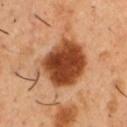Clinical impression: Imaged during a routine full-body skin examination; the lesion was not biopsied and no histopathology is available. Image and clinical context: The recorded lesion diameter is about 6.5 mm. Imaged with cross-polarized lighting. Cropped from a total-body skin-imaging series; the visible field is about 15 mm. Located on the chest. A male subject, in their 50s.